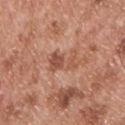Recorded during total-body skin imaging; not selected for excision or biopsy. The patient is a male aged 53–57. Captured under white-light illumination. The lesion is located on the upper back. Cropped from a whole-body photographic skin survey; the tile spans about 15 mm.